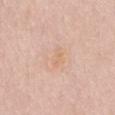{"biopsy_status": "not biopsied; imaged during a skin examination"}